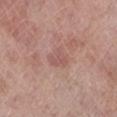No biopsy was performed on this lesion — it was imaged during a full skin examination and was not determined to be concerning. The recorded lesion diameter is about 3 mm. Located on the left lower leg. An algorithmic analysis of the crop reported a lesion area of about 5 mm², an outline eccentricity of about 0.45 (0 = round, 1 = elongated), and a symmetry-axis asymmetry near 0.4. A female subject about 70 years old. A 15 mm close-up tile from a total-body photography series done for melanoma screening.